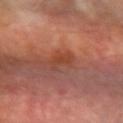<lesion>
<biopsy_status>not biopsied; imaged during a skin examination</biopsy_status>
<patient>
  <sex>male</sex>
  <age_approx>70</age_approx>
</patient>
<lighting>cross-polarized</lighting>
<site>left forearm</site>
<image>
  <source>total-body photography crop</source>
  <field_of_view_mm>15</field_of_view_mm>
</image>
<lesion_size>
  <long_diameter_mm_approx>3.0</long_diameter_mm_approx>
</lesion_size>
</lesion>